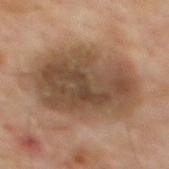  biopsy_status: not biopsied; imaged during a skin examination
  site: mid back
  patient:
    sex: male
    age_approx: 65
  image:
    source: total-body photography crop
    field_of_view_mm: 15
  lesion_size:
    long_diameter_mm_approx: 10.0